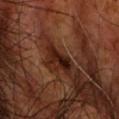Notes:
- workup · imaged on a skin check; not biopsied
- acquisition · 15 mm crop, total-body photography
- lighting · cross-polarized
- site · the head or neck
- lesion diameter · ~4.5 mm (longest diameter)
- subject · male, aged 68–72